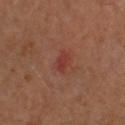The subject is a male aged 63–67.
The recorded lesion diameter is about 2.5 mm.
The lesion is on the left forearm.
The total-body-photography lesion software estimated a footprint of about 3 mm², an outline eccentricity of about 0.85 (0 = round, 1 = elongated), and a symmetry-axis asymmetry near 0.35. And it measured border irregularity of about 3 on a 0–10 scale and a within-lesion color-variation index near 1/10.
A roughly 15 mm field-of-view crop from a total-body skin photograph.
The tile uses cross-polarized illumination.